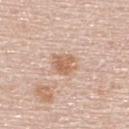Case summary:
- follow-up: imaged on a skin check; not biopsied
- lesion size: ≈3 mm
- subject: male, aged approximately 60
- location: the upper back
- image source: 15 mm crop, total-body photography